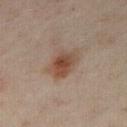Part of a total-body skin-imaging series; this lesion was reviewed on a skin check and was not flagged for biopsy.
This is a cross-polarized tile.
The lesion is on the right thigh.
The lesion's longest dimension is about 4 mm.
The total-body-photography lesion software estimated a lesion color around L≈45 a*≈18 b*≈26 in CIELAB, roughly 10 lightness units darker than nearby skin, and a normalized border contrast of about 8.5.
The patient is a female aged 33–37.
A 15 mm close-up tile from a total-body photography series done for melanoma screening.